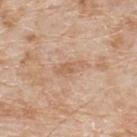workup — catalogued during a skin exam; not biopsied | diameter — ~3.5 mm (longest diameter) | body site — the upper back | acquisition — ~15 mm crop, total-body skin-cancer survey | patient — male, about 80 years old | image-analysis metrics — a footprint of about 3.5 mm² and an outline eccentricity of about 0.95 (0 = round, 1 = elongated); a lesion color around L≈61 a*≈19 b*≈33 in CIELAB, about 8 CIELAB-L* units darker than the surrounding skin, and a lesion-to-skin contrast of about 6 (normalized; higher = more distinct); a nevus-likeness score of about 0/100 and a detector confidence of about 100 out of 100 that the crop contains a lesion.